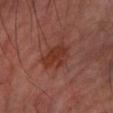<case>
<biopsy_status>not biopsied; imaged during a skin examination</biopsy_status>
<site>left forearm</site>
<automated_metrics>
  <border_irregularity_0_10>3.0</border_irregularity_0_10>
  <color_variation_0_10>3.0</color_variation_0_10>
  <peripheral_color_asymmetry>1.0</peripheral_color_asymmetry>
  <lesion_detection_confidence_0_100>100</lesion_detection_confidence_0_100>
</automated_metrics>
<lesion_size>
  <long_diameter_mm_approx>4.0</long_diameter_mm_approx>
</lesion_size>
<lighting>cross-polarized</lighting>
<patient>
  <sex>male</sex>
  <age_approx>60</age_approx>
</patient>
<image>
  <source>total-body photography crop</source>
  <field_of_view_mm>15</field_of_view_mm>
</image>
</case>